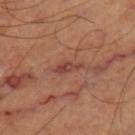Q: Is there a histopathology result?
A: no biopsy performed (imaged during a skin exam)
Q: Patient demographics?
A: in their mid-60s
Q: Where on the body is the lesion?
A: the right thigh
Q: Lesion size?
A: ≈3 mm
Q: What kind of image is this?
A: ~15 mm tile from a whole-body skin photo